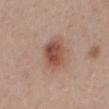The lesion was photographed on a routine skin check and not biopsied; there is no pathology result.
The patient is a female roughly 40 years of age.
Located on the mid back.
Cropped from a total-body skin-imaging series; the visible field is about 15 mm.